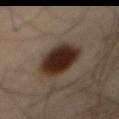<case>
<biopsy_status>not biopsied; imaged during a skin examination</biopsy_status>
<lighting>cross-polarized</lighting>
<lesion_size>
  <long_diameter_mm_approx>5.0</long_diameter_mm_approx>
</lesion_size>
<patient>
  <sex>male</sex>
  <age_approx>55</age_approx>
</patient>
<automated_metrics>
  <border_irregularity_0_10>1.5</border_irregularity_0_10>
  <peripheral_color_asymmetry>1.0</peripheral_color_asymmetry>
  <nevus_likeness_0_100>100</nevus_likeness_0_100>
  <lesion_detection_confidence_0_100>100</lesion_detection_confidence_0_100>
</automated_metrics>
<site>abdomen</site>
<image>
  <source>total-body photography crop</source>
  <field_of_view_mm>15</field_of_view_mm>
</image>
</case>